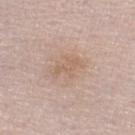Q: Was a biopsy performed?
A: catalogued during a skin exam; not biopsied
Q: Lesion location?
A: the right lower leg
Q: How was this image acquired?
A: ~15 mm crop, total-body skin-cancer survey
Q: How was the tile lit?
A: white-light illumination
Q: Automated lesion metrics?
A: an eccentricity of roughly 0.85 and two-axis asymmetry of about 0.55; a lesion color around L≈61 a*≈17 b*≈28 in CIELAB and a lesion–skin lightness drop of about 6; a border-irregularity rating of about 7.5/10, a within-lesion color-variation index near 0/10, and peripheral color asymmetry of about 0
Q: What is the lesion's diameter?
A: about 3 mm
Q: What are the patient's age and sex?
A: male, about 65 years old